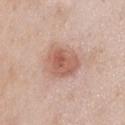Q: Was this lesion biopsied?
A: catalogued during a skin exam; not biopsied
Q: Lesion size?
A: ≈5 mm
Q: Illumination type?
A: white-light illumination
Q: How was this image acquired?
A: ~15 mm crop, total-body skin-cancer survey
Q: Patient demographics?
A: male, about 55 years old
Q: Where on the body is the lesion?
A: the front of the torso
Q: Automated lesion metrics?
A: a footprint of about 13 mm², an outline eccentricity of about 0.65 (0 = round, 1 = elongated), and a shape-asymmetry score of about 0.2 (0 = symmetric); a lesion color around L≈59 a*≈22 b*≈28 in CIELAB; a classifier nevus-likeness of about 85/100 and a detector confidence of about 100 out of 100 that the crop contains a lesion